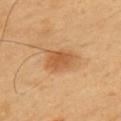  biopsy_status: not biopsied; imaged during a skin examination
  lesion_size:
    long_diameter_mm_approx: 4.0
  site: left upper arm
  patient:
    sex: male
    age_approx: 55
  image:
    source: total-body photography crop
    field_of_view_mm: 15